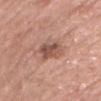Notes:
– follow-up — imaged on a skin check; not biopsied
– size — ~3 mm (longest diameter)
– lighting — white-light illumination
– image — total-body-photography crop, ~15 mm field of view
– body site — the head or neck
– subject — female, aged around 70